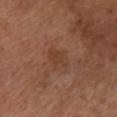workup: imaged on a skin check; not biopsied | lesion size: about 3 mm | lighting: cross-polarized illumination | subject: male, aged 63–67 | site: the left lower leg | image: 15 mm crop, total-body photography.